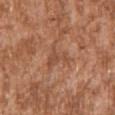Q: Was this lesion biopsied?
A: imaged on a skin check; not biopsied
Q: Lesion location?
A: the arm
Q: How was the tile lit?
A: white-light
Q: Who is the patient?
A: male, aged 43–47
Q: What is the imaging modality?
A: total-body-photography crop, ~15 mm field of view
Q: How large is the lesion?
A: ~3.5 mm (longest diameter)